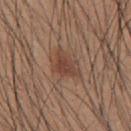{"biopsy_status": "not biopsied; imaged during a skin examination", "site": "upper back", "patient": {"sex": "male", "age_approx": 20}, "image": {"source": "total-body photography crop", "field_of_view_mm": 15}}